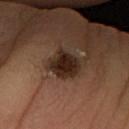Q: Was this lesion biopsied?
A: imaged on a skin check; not biopsied
Q: Automated lesion metrics?
A: a mean CIELAB color near L≈24 a*≈16 b*≈23, a lesion–skin lightness drop of about 13, and a normalized lesion–skin contrast near 13; border irregularity of about 2 on a 0–10 scale, a within-lesion color-variation index near 4/10, and radial color variation of about 1; a classifier nevus-likeness of about 80/100 and a lesion-detection confidence of about 100/100
Q: What are the patient's age and sex?
A: male, in their mid- to late 60s
Q: What is the anatomic site?
A: the left forearm
Q: What kind of image is this?
A: ~15 mm tile from a whole-body skin photo